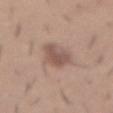The lesion was photographed on a routine skin check and not biopsied; there is no pathology result.
A 15 mm crop from a total-body photograph taken for skin-cancer surveillance.
A male subject, aged 28–32.
The tile uses white-light illumination.
Automated tile analysis of the lesion measured roughly 10 lightness units darker than nearby skin and a normalized lesion–skin contrast near 7.5. And it measured a nevus-likeness score of about 30/100 and a detector confidence of about 100 out of 100 that the crop contains a lesion.
Measured at roughly 5 mm in maximum diameter.
The lesion is located on the front of the torso.